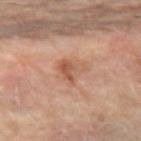Case summary:
* biopsy status — no biopsy performed (imaged during a skin exam)
* acquisition — ~15 mm tile from a whole-body skin photo
* subject — female, aged 63–67
* site — the left forearm